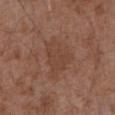Notes:
- notes — no biopsy performed (imaged during a skin exam)
- lesion size — ~5.5 mm (longest diameter)
- subject — male, about 75 years old
- TBP lesion metrics — a normalized lesion–skin contrast near 4.5; a border-irregularity rating of about 4.5/10, a color-variation rating of about 2/10, and a peripheral color-asymmetry measure near 0.5
- imaging modality — ~15 mm tile from a whole-body skin photo
- illumination — white-light
- anatomic site — the abdomen